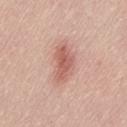biopsy status: catalogued during a skin exam; not biopsied | image source: 15 mm crop, total-body photography | patient: female, aged 63–67 | site: the lower back.